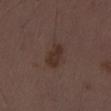| feature | finding |
|---|---|
| biopsy status | total-body-photography surveillance lesion; no biopsy |
| image | total-body-photography crop, ~15 mm field of view |
| automated lesion analysis | an automated nevus-likeness rating near 25 out of 100 and a lesion-detection confidence of about 100/100 |
| lighting | white-light illumination |
| patient | male, aged 28 to 32 |
| body site | the left thigh |